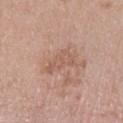Impression:
The lesion was photographed on a routine skin check and not biopsied; there is no pathology result.
Clinical summary:
A female subject, aged 68–72. The lesion is located on the left lower leg. A 15 mm close-up extracted from a 3D total-body photography capture. The tile uses white-light illumination. The lesion's longest dimension is about 3.5 mm.